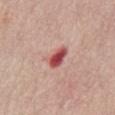The lesion was tiled from a total-body skin photograph and was not biopsied.